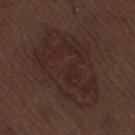Clinical impression: Captured during whole-body skin photography for melanoma surveillance; the lesion was not biopsied. Clinical summary: This is a white-light tile. Cropped from a whole-body photographic skin survey; the tile spans about 15 mm. Measured at roughly 9.5 mm in maximum diameter. A male subject, approximately 70 years of age. The lesion is on the right thigh. The lesion-visualizer software estimated an automated nevus-likeness rating near 10 out of 100 and lesion-presence confidence of about 100/100.